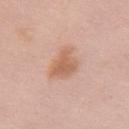Recorded during total-body skin imaging; not selected for excision or biopsy.
The lesion is located on the chest.
A roughly 15 mm field-of-view crop from a total-body skin photograph.
A female subject aged approximately 65.